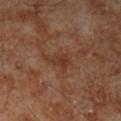<case>
  <biopsy_status>not biopsied; imaged during a skin examination</biopsy_status>
  <patient>
    <sex>male</sex>
    <age_approx>70</age_approx>
  </patient>
  <image>
    <source>total-body photography crop</source>
    <field_of_view_mm>15</field_of_view_mm>
  </image>
  <site>leg</site>
</case>